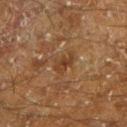notes — imaged on a skin check; not biopsied | tile lighting — cross-polarized illumination | imaging modality — ~15 mm crop, total-body skin-cancer survey | body site — the left lower leg | patient — male, aged 58 to 62 | automated lesion analysis — a lesion color around L≈30 a*≈16 b*≈26 in CIELAB, about 6 CIELAB-L* units darker than the surrounding skin, and a normalized border contrast of about 6.5; border irregularity of about 4 on a 0–10 scale and a within-lesion color-variation index near 2/10; a classifier nevus-likeness of about 0/100 and a lesion-detection confidence of about 75/100.